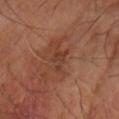No biopsy was performed on this lesion — it was imaged during a full skin examination and was not determined to be concerning. A male patient about 70 years old. A 15 mm crop from a total-body photograph taken for skin-cancer surveillance. Located on the right forearm. The lesion's longest dimension is about 3 mm.